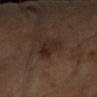Notes:
* follow-up — imaged on a skin check; not biopsied
* patient — female, aged 58–62
* acquisition — total-body-photography crop, ~15 mm field of view
* location — the left forearm
* size — ~3.5 mm (longest diameter)